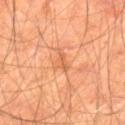Q: Was a biopsy performed?
A: no biopsy performed (imaged during a skin exam)
Q: How large is the lesion?
A: about 3 mm
Q: Patient demographics?
A: male, aged approximately 65
Q: What is the anatomic site?
A: the left thigh
Q: Automated lesion metrics?
A: a shape eccentricity near 0.9
Q: What lighting was used for the tile?
A: cross-polarized
Q: What kind of image is this?
A: ~15 mm tile from a whole-body skin photo